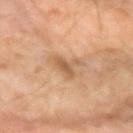notes = no biopsy performed (imaged during a skin exam)
lesion size = about 3.5 mm
image = ~15 mm tile from a whole-body skin photo
tile lighting = cross-polarized
location = the left forearm
subject = male, about 60 years old
TBP lesion metrics = a footprint of about 5.5 mm², a shape eccentricity near 0.4, and a symmetry-axis asymmetry near 0.6; lesion-presence confidence of about 100/100